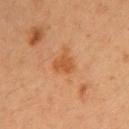| key | value |
|---|---|
| acquisition | ~15 mm tile from a whole-body skin photo |
| patient | female, aged 38–42 |
| illumination | cross-polarized illumination |
| location | the left upper arm |